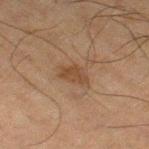Assessment: Recorded during total-body skin imaging; not selected for excision or biopsy. Acquisition and patient details: Automated tile analysis of the lesion measured a mean CIELAB color near L≈37 a*≈16 b*≈28. The software also gave border irregularity of about 3 on a 0–10 scale, a within-lesion color-variation index near 2/10, and a peripheral color-asymmetry measure near 0.5. From the right thigh. A roughly 15 mm field-of-view crop from a total-body skin photograph. The patient is a male aged approximately 65. The lesion's longest dimension is about 3 mm. Imaged with cross-polarized lighting.